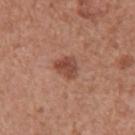This lesion was catalogued during total-body skin photography and was not selected for biopsy.
This image is a 15 mm lesion crop taken from a total-body photograph.
Captured under white-light illumination.
The patient is a male approximately 45 years of age.
The lesion-visualizer software estimated an average lesion color of about L≈47 a*≈24 b*≈29 (CIELAB), about 11 CIELAB-L* units darker than the surrounding skin, and a lesion-to-skin contrast of about 8 (normalized; higher = more distinct). The software also gave internal color variation of about 3.5 on a 0–10 scale and peripheral color asymmetry of about 1.5.
From the arm.
Longest diameter approximately 3 mm.